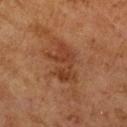imaging modality = total-body-photography crop, ~15 mm field of view | site = the left upper arm | patient = female, about 60 years old | illumination = cross-polarized illumination.